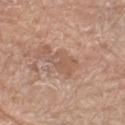<record>
<biopsy_status>not biopsied; imaged during a skin examination</biopsy_status>
<automated_metrics>
  <area_mm2_approx>5.0</area_mm2_approx>
  <eccentricity>0.8</eccentricity>
  <shape_asymmetry>0.4</shape_asymmetry>
  <color_variation_0_10>2.0</color_variation_0_10>
  <peripheral_color_asymmetry>0.5</peripheral_color_asymmetry>
</automated_metrics>
<site>leg</site>
<patient>
  <sex>female</sex>
  <age_approx>75</age_approx>
</patient>
<lighting>white-light</lighting>
<lesion_size>
  <long_diameter_mm_approx>3.5</long_diameter_mm_approx>
</lesion_size>
<image>
  <source>total-body photography crop</source>
  <field_of_view_mm>15</field_of_view_mm>
</image>
</record>